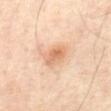site: the front of the torso | image: ~15 mm crop, total-body skin-cancer survey | patient: male, aged approximately 55 | TBP lesion metrics: a lesion area of about 7 mm², an eccentricity of roughly 0.7, and a shape-asymmetry score of about 0.2 (0 = symmetric); a border-irregularity rating of about 2/10, a within-lesion color-variation index near 4.5/10, and radial color variation of about 1.5; a classifier nevus-likeness of about 70/100 and a lesion-detection confidence of about 100/100 | size: ≈3.5 mm.